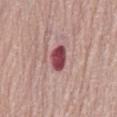Captured during whole-body skin photography for melanoma surveillance; the lesion was not biopsied. A 15 mm close-up extracted from a 3D total-body photography capture. The lesion's longest dimension is about 3.5 mm. The patient is a female in their mid-60s. On the front of the torso. Captured under white-light illumination.